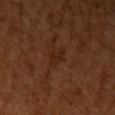The lesion was tiled from a total-body skin photograph and was not biopsied. Automated tile analysis of the lesion measured an area of roughly 3 mm² and two-axis asymmetry of about 0.45. The software also gave an average lesion color of about L≈24 a*≈20 b*≈28 (CIELAB), a lesion–skin lightness drop of about 5, and a normalized lesion–skin contrast near 6. The software also gave border irregularity of about 5 on a 0–10 scale. It also reported a classifier nevus-likeness of about 5/100 and lesion-presence confidence of about 95/100. Longest diameter approximately 3 mm. On the left upper arm. A close-up tile cropped from a whole-body skin photograph, about 15 mm across. A female subject, aged 53 to 57.